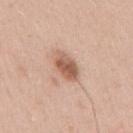Recorded during total-body skin imaging; not selected for excision or biopsy. The patient is a male aged 38 to 42. A close-up tile cropped from a whole-body skin photograph, about 15 mm across. The recorded lesion diameter is about 4 mm. On the right upper arm.